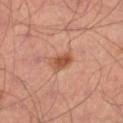Findings:
• workup · imaged on a skin check; not biopsied
• site · the left leg
• patient · male, aged around 35
• image source · 15 mm crop, total-body photography
• illumination · cross-polarized illumination